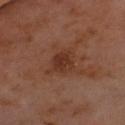| feature | finding |
|---|---|
| follow-up | catalogued during a skin exam; not biopsied |
| illumination | cross-polarized illumination |
| anatomic site | the front of the torso |
| diameter | about 3.5 mm |
| imaging modality | 15 mm crop, total-body photography |
| image-analysis metrics | an average lesion color of about L≈34 a*≈22 b*≈28 (CIELAB), about 8 CIELAB-L* units darker than the surrounding skin, and a normalized lesion–skin contrast near 7.5; a classifier nevus-likeness of about 15/100 and a lesion-detection confidence of about 100/100 |
| patient | male, in their mid- to late 50s |